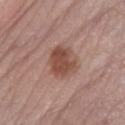This lesion was catalogued during total-body skin photography and was not selected for biopsy.
A 15 mm close-up tile from a total-body photography series done for melanoma screening.
The lesion is located on the left lower leg.
The tile uses white-light illumination.
The subject is a female roughly 50 years of age.
The total-body-photography lesion software estimated a footprint of about 11 mm², an eccentricity of roughly 0.55, and a symmetry-axis asymmetry near 0.15. And it measured an average lesion color of about L≈48 a*≈22 b*≈26 (CIELAB), about 11 CIELAB-L* units darker than the surrounding skin, and a normalized border contrast of about 8.5. It also reported an automated nevus-likeness rating near 80 out of 100 and a detector confidence of about 100 out of 100 that the crop contains a lesion.
The recorded lesion diameter is about 4 mm.